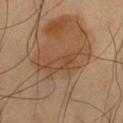– size — ~7 mm (longest diameter)
– site — the left thigh
– imaging modality — ~15 mm tile from a whole-body skin photo
– patient — male, aged approximately 60